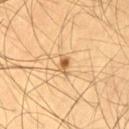Q: Was this lesion biopsied?
A: catalogued during a skin exam; not biopsied
Q: What is the lesion's diameter?
A: about 2.5 mm
Q: What lighting was used for the tile?
A: cross-polarized
Q: Automated lesion metrics?
A: an area of roughly 3 mm², an eccentricity of roughly 0.8, and two-axis asymmetry of about 0.3; border irregularity of about 3 on a 0–10 scale, a within-lesion color-variation index near 5.5/10, and peripheral color asymmetry of about 1.5; lesion-presence confidence of about 100/100
Q: Patient demographics?
A: male, aged 53–57
Q: What is the imaging modality?
A: ~15 mm crop, total-body skin-cancer survey
Q: Where on the body is the lesion?
A: the leg